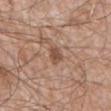No biopsy was performed on this lesion — it was imaged during a full skin examination and was not determined to be concerning. Longest diameter approximately 2.5 mm. A male patient approximately 60 years of age. This is a white-light tile. Cropped from a total-body skin-imaging series; the visible field is about 15 mm. The lesion is on the mid back.